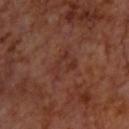No biopsy was performed on this lesion — it was imaged during a full skin examination and was not determined to be concerning.
A lesion tile, about 15 mm wide, cut from a 3D total-body photograph.
The lesion is located on the upper back.
A male patient, aged around 70.
Captured under cross-polarized illumination.